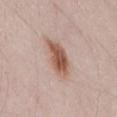Assessment: Part of a total-body skin-imaging series; this lesion was reviewed on a skin check and was not flagged for biopsy. Acquisition and patient details: The lesion's longest dimension is about 5 mm. A male patient, aged 23–27. Automated image analysis of the tile measured an area of roughly 10 mm², an eccentricity of roughly 0.8, and a symmetry-axis asymmetry near 0.2. It also reported about 13 CIELAB-L* units darker than the surrounding skin and a normalized lesion–skin contrast near 9.5. The software also gave border irregularity of about 2.5 on a 0–10 scale, a within-lesion color-variation index near 4.5/10, and a peripheral color-asymmetry measure near 1.5. The analysis additionally found a classifier nevus-likeness of about 100/100 and lesion-presence confidence of about 100/100. The lesion is located on the lower back. A roughly 15 mm field-of-view crop from a total-body skin photograph. Imaged with white-light lighting.